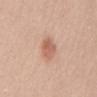Part of a total-body skin-imaging series; this lesion was reviewed on a skin check and was not flagged for biopsy. On the back. Captured under white-light illumination. A female patient, aged 38 to 42. A 15 mm close-up extracted from a 3D total-body photography capture. An algorithmic analysis of the crop reported a footprint of about 6.5 mm² and an eccentricity of roughly 0.6. And it measured an average lesion color of about L≈61 a*≈23 b*≈30 (CIELAB), a lesion–skin lightness drop of about 10, and a normalized border contrast of about 6.5. The software also gave a border-irregularity index near 1.5/10 and a within-lesion color-variation index near 3/10. The analysis additionally found an automated nevus-likeness rating near 90 out of 100 and a detector confidence of about 100 out of 100 that the crop contains a lesion.